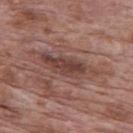No biopsy was performed on this lesion — it was imaged during a full skin examination and was not determined to be concerning.
The patient is a male roughly 70 years of age.
From the mid back.
Imaged with white-light lighting.
Automated tile analysis of the lesion measured an average lesion color of about L≈43 a*≈20 b*≈22 (CIELAB) and about 10 CIELAB-L* units darker than the surrounding skin. The analysis additionally found border irregularity of about 4 on a 0–10 scale and radial color variation of about 1.5.
Measured at roughly 7 mm in maximum diameter.
A lesion tile, about 15 mm wide, cut from a 3D total-body photograph.